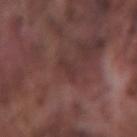No biopsy was performed on this lesion — it was imaged during a full skin examination and was not determined to be concerning.
A close-up tile cropped from a whole-body skin photograph, about 15 mm across.
Longest diameter approximately 3 mm.
Imaged with white-light lighting.
Located on the left forearm.
The subject is a male roughly 75 years of age.